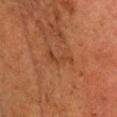Assessment:
The lesion was tiled from a total-body skin photograph and was not biopsied.
Clinical summary:
The lesion's longest dimension is about 4 mm. The tile uses cross-polarized illumination. A male subject approximately 50 years of age. The total-body-photography lesion software estimated a lesion area of about 3.5 mm² and an eccentricity of roughly 0.95. The analysis additionally found a lesion color around L≈32 a*≈20 b*≈29 in CIELAB and a normalized border contrast of about 5. And it measured a border-irregularity index near 7.5/10, a color-variation rating of about 0/10, and radial color variation of about 0. A lesion tile, about 15 mm wide, cut from a 3D total-body photograph. From the head or neck.